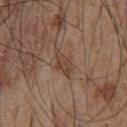Background:
The lesion is on the chest. Captured under white-light illumination. Approximately 3 mm at its widest. Automated image analysis of the tile measured a shape eccentricity near 0.85 and a symmetry-axis asymmetry near 0.25. It also reported a mean CIELAB color near L≈43 a*≈17 b*≈27, a lesion–skin lightness drop of about 8, and a normalized lesion–skin contrast near 6.5. The software also gave a detector confidence of about 75 out of 100 that the crop contains a lesion. A male patient aged 53–57. A region of skin cropped from a whole-body photographic capture, roughly 15 mm wide.